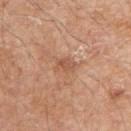From the right upper arm. The subject is a male in their 80s. The recorded lesion diameter is about 2.5 mm. Imaged with white-light lighting. The total-body-photography lesion software estimated an area of roughly 3.5 mm², an outline eccentricity of about 0.75 (0 = round, 1 = elongated), and a shape-asymmetry score of about 0.35 (0 = symmetric). The analysis additionally found a classifier nevus-likeness of about 0/100. A 15 mm close-up tile from a total-body photography series done for melanoma screening.